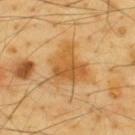Q: Was this lesion biopsied?
A: no biopsy performed (imaged during a skin exam)
Q: Lesion location?
A: the upper back
Q: What are the patient's age and sex?
A: male, about 65 years old
Q: What is the imaging modality?
A: 15 mm crop, total-body photography